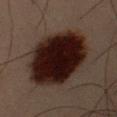| feature | finding |
|---|---|
| notes | no biopsy performed (imaged during a skin exam) |
| patient | male, aged 48 to 52 |
| lesion diameter | ~9.5 mm (longest diameter) |
| illumination | cross-polarized |
| imaging modality | total-body-photography crop, ~15 mm field of view |
| anatomic site | the left upper arm |